A 15 mm close-up extracted from a 3D total-body photography capture · the lesion is located on the left upper arm · Automated tile analysis of the lesion measured a shape eccentricity near 0.6 and two-axis asymmetry of about 0.15. The software also gave internal color variation of about 4 on a 0–10 scale and a peripheral color-asymmetry measure near 1. The software also gave lesion-presence confidence of about 100/100 · captured under white-light illumination · a female subject, roughly 65 years of age · the lesion's longest dimension is about 5.5 mm:
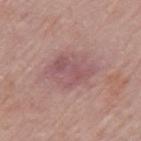Histopathological examination showed a dermatofibroma.